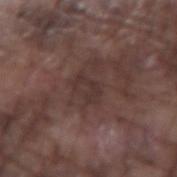Case summary:
• follow-up: total-body-photography surveillance lesion; no biopsy
• automated lesion analysis: a classifier nevus-likeness of about 0/100 and a lesion-detection confidence of about 95/100
• patient: male, aged approximately 75
• lesion diameter: ≈3 mm
• imaging modality: total-body-photography crop, ~15 mm field of view
• body site: the right forearm